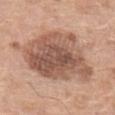Q: Is there a histopathology result?
A: catalogued during a skin exam; not biopsied
Q: Lesion location?
A: the left thigh
Q: What are the patient's age and sex?
A: female, aged 58–62
Q: What kind of image is this?
A: ~15 mm tile from a whole-body skin photo
Q: How was the tile lit?
A: white-light illumination
Q: Lesion size?
A: ≈10 mm